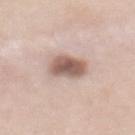No biopsy was performed on this lesion — it was imaged during a full skin examination and was not determined to be concerning.
The subject is a female approximately 50 years of age.
From the back.
Captured under white-light illumination.
A 15 mm close-up tile from a total-body photography series done for melanoma screening.
The total-body-photography lesion software estimated an area of roughly 9 mm², an eccentricity of roughly 0.3, and a shape-asymmetry score of about 0.2 (0 = symmetric). The analysis additionally found an average lesion color of about L≈57 a*≈18 b*≈24 (CIELAB), roughly 16 lightness units darker than nearby skin, and a normalized lesion–skin contrast near 10. The analysis additionally found a nevus-likeness score of about 75/100.